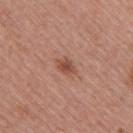A female patient, aged approximately 55. The recorded lesion diameter is about 2.5 mm. The lesion is located on the right upper arm. This is a white-light tile. A region of skin cropped from a whole-body photographic capture, roughly 15 mm wide.